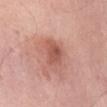Assessment: Recorded during total-body skin imaging; not selected for excision or biopsy. Clinical summary: This is a white-light tile. About 3 mm across. The patient is a female approximately 50 years of age. On the abdomen. This image is a 15 mm lesion crop taken from a total-body photograph.